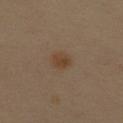| key | value |
|---|---|
| notes | total-body-photography surveillance lesion; no biopsy |
| location | the back |
| image-analysis metrics | a shape eccentricity near 0.75 and a symmetry-axis asymmetry near 0.2; a detector confidence of about 100 out of 100 that the crop contains a lesion |
| patient | female, aged around 60 |
| image | ~15 mm tile from a whole-body skin photo |
| tile lighting | cross-polarized illumination |
| diameter | about 2.5 mm |